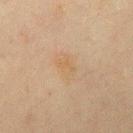notes: total-body-photography surveillance lesion; no biopsy
body site: the chest
automated lesion analysis: a border-irregularity rating of about 2.5/10 and radial color variation of about 0.5
subject: female, approximately 50 years of age
image source: ~15 mm tile from a whole-body skin photo